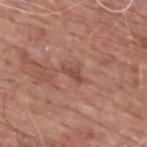Recorded during total-body skin imaging; not selected for excision or biopsy. The lesion-visualizer software estimated a lesion area of about 3 mm² and two-axis asymmetry of about 0.4. It also reported a lesion-to-skin contrast of about 6.5 (normalized; higher = more distinct). The software also gave border irregularity of about 4 on a 0–10 scale and a color-variation rating of about 1/10. A male subject aged approximately 60. About 3 mm across. A 15 mm close-up tile from a total-body photography series done for melanoma screening. The lesion is on the upper back. Captured under white-light illumination.